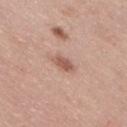  biopsy_status: not biopsied; imaged during a skin examination
  lesion_size:
    long_diameter_mm_approx: 3.0
  lighting: white-light
  image:
    source: total-body photography crop
    field_of_view_mm: 15
  patient:
    sex: female
    age_approx: 40
  site: right thigh